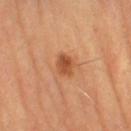Q: Was this lesion biopsied?
A: imaged on a skin check; not biopsied
Q: What is the lesion's diameter?
A: ≈2.5 mm
Q: What is the anatomic site?
A: the left thigh
Q: What are the patient's age and sex?
A: female, in their mid-60s
Q: Automated lesion metrics?
A: a footprint of about 4.5 mm²; an average lesion color of about L≈50 a*≈27 b*≈37 (CIELAB), about 11 CIELAB-L* units darker than the surrounding skin, and a normalized border contrast of about 8; a classifier nevus-likeness of about 85/100 and lesion-presence confidence of about 100/100
Q: What lighting was used for the tile?
A: cross-polarized
Q: What kind of image is this?
A: ~15 mm crop, total-body skin-cancer survey